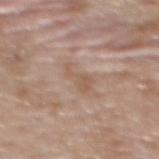image source — total-body-photography crop, ~15 mm field of view
patient — female, aged around 60
body site — the upper back